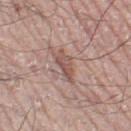Imaged during a routine full-body skin examination; the lesion was not biopsied and no histopathology is available. A lesion tile, about 15 mm wide, cut from a 3D total-body photograph. Located on the right leg. The subject is a male in their 70s.